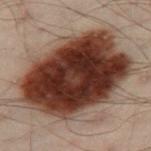| field | value |
|---|---|
| follow-up | catalogued during a skin exam; not biopsied |
| patient | male, roughly 50 years of age |
| image-analysis metrics | a border-irregularity index near 2/10 and a within-lesion color-variation index near 7.5/10; a classifier nevus-likeness of about 100/100 and a detector confidence of about 100 out of 100 that the crop contains a lesion |
| illumination | cross-polarized illumination |
| body site | the left leg |
| lesion diameter | about 11.5 mm |
| image source | ~15 mm tile from a whole-body skin photo |